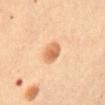Acquisition and patient details:
The total-body-photography lesion software estimated an area of roughly 5.5 mm², an outline eccentricity of about 0.6 (0 = round, 1 = elongated), and a symmetry-axis asymmetry near 0.2. The analysis additionally found an average lesion color of about L≈65 a*≈24 b*≈39 (CIELAB), roughly 13 lightness units darker than nearby skin, and a normalized border contrast of about 8. And it measured a border-irregularity index near 2/10 and peripheral color asymmetry of about 1. The analysis additionally found a classifier nevus-likeness of about 100/100 and a detector confidence of about 100 out of 100 that the crop contains a lesion. A 15 mm close-up tile from a total-body photography series done for melanoma screening. The lesion is on the abdomen. A female patient, aged 63 to 67. Imaged with cross-polarized lighting. The lesion's longest dimension is about 3 mm.